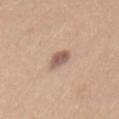follow-up=catalogued during a skin exam; not biopsied
acquisition=~15 mm tile from a whole-body skin photo
subject=female, aged 33–37
lesion size=≈3 mm
illumination=white-light
location=the mid back